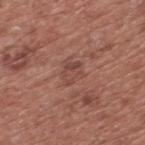Case summary:
– biopsy status — imaged on a skin check; not biopsied
– diameter — about 3 mm
– lighting — white-light illumination
– subject — male, about 75 years old
– image — ~15 mm crop, total-body skin-cancer survey
– site — the back
– TBP lesion metrics — a mean CIELAB color near L≈45 a*≈23 b*≈24, a lesion–skin lightness drop of about 7, and a normalized border contrast of about 5.5; a border-irregularity rating of about 3/10, a within-lesion color-variation index near 4.5/10, and peripheral color asymmetry of about 2